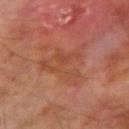Impression:
Captured during whole-body skin photography for melanoma surveillance; the lesion was not biopsied.
Image and clinical context:
The lesion's longest dimension is about 6 mm. The total-body-photography lesion software estimated a lesion–skin lightness drop of about 5 and a lesion-to-skin contrast of about 5 (normalized; higher = more distinct). A male subject, about 70 years old. The lesion is located on the right upper arm. This image is a 15 mm lesion crop taken from a total-body photograph.